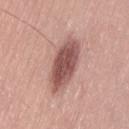Clinical impression: This lesion was catalogued during total-body skin photography and was not selected for biopsy. Clinical summary: The lesion is located on the lower back. Automated tile analysis of the lesion measured an area of roughly 17 mm², a shape eccentricity near 0.9, and a symmetry-axis asymmetry near 0.15. And it measured internal color variation of about 4.5 on a 0–10 scale and a peripheral color-asymmetry measure near 1.5. A male patient aged approximately 25. Imaged with white-light lighting. Longest diameter approximately 7 mm. A roughly 15 mm field-of-view crop from a total-body skin photograph.